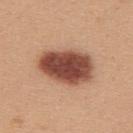Q: Was this lesion biopsied?
A: imaged on a skin check; not biopsied
Q: What are the patient's age and sex?
A: female, aged 23 to 27
Q: What kind of image is this?
A: total-body-photography crop, ~15 mm field of view
Q: Where on the body is the lesion?
A: the upper back
Q: Automated lesion metrics?
A: an area of roughly 23 mm² and an outline eccentricity of about 0.8 (0 = round, 1 = elongated); an average lesion color of about L≈48 a*≈24 b*≈29 (CIELAB) and a lesion-to-skin contrast of about 13 (normalized; higher = more distinct); border irregularity of about 1.5 on a 0–10 scale and a peripheral color-asymmetry measure near 2; a nevus-likeness score of about 100/100 and a lesion-detection confidence of about 100/100